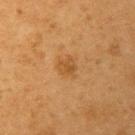size=~3 mm (longest diameter)
TBP lesion metrics=a lesion color around L≈47 a*≈20 b*≈39 in CIELAB, roughly 7 lightness units darker than nearby skin, and a normalized border contrast of about 5.5; lesion-presence confidence of about 100/100
tile lighting=cross-polarized
location=the left upper arm
patient=male, aged approximately 55
image source=15 mm crop, total-body photography